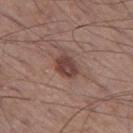A 15 mm close-up tile from a total-body photography series done for melanoma screening.
This is a white-light tile.
From the left thigh.
The lesion's longest dimension is about 3 mm.
A male subject aged 58 to 62.
An algorithmic analysis of the crop reported a lesion color around L≈42 a*≈20 b*≈22 in CIELAB and a normalized border contrast of about 9. The analysis additionally found border irregularity of about 2 on a 0–10 scale, a within-lesion color-variation index near 3/10, and a peripheral color-asymmetry measure near 1. The analysis additionally found an automated nevus-likeness rating near 80 out of 100.